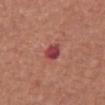• notes: catalogued during a skin exam; not biopsied